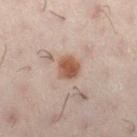• biopsy status: total-body-photography surveillance lesion; no biopsy
• image source: total-body-photography crop, ~15 mm field of view
• patient: female, in their 30s
• location: the left leg